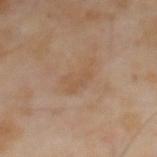Findings:
- notes: no biopsy performed (imaged during a skin exam)
- illumination: cross-polarized
- patient: male, aged 63–67
- image source: total-body-photography crop, ~15 mm field of view
- automated lesion analysis: a lesion area of about 6.5 mm² and a shape-asymmetry score of about 0.45 (0 = symmetric); roughly 5 lightness units darker than nearby skin and a lesion-to-skin contrast of about 4.5 (normalized; higher = more distinct)